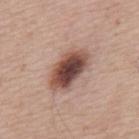| key | value |
|---|---|
| workup | no biopsy performed (imaged during a skin exam) |
| lighting | white-light illumination |
| anatomic site | the mid back |
| TBP lesion metrics | an area of roughly 15 mm² and a shape eccentricity near 0.85; a border-irregularity rating of about 2/10, a color-variation rating of about 7.5/10, and peripheral color asymmetry of about 1.5 |
| size | ≈6 mm |
| patient | male, aged approximately 65 |
| imaging modality | ~15 mm tile from a whole-body skin photo |